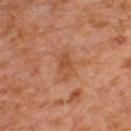Case summary:
* notes · total-body-photography surveillance lesion; no biopsy
* tile lighting · cross-polarized illumination
* lesion diameter · ≈3.5 mm
* patient · male, about 30 years old
* anatomic site · the leg
* TBP lesion metrics · a lesion color around L≈49 a*≈24 b*≈35 in CIELAB and a lesion-to-skin contrast of about 6 (normalized; higher = more distinct); border irregularity of about 2.5 on a 0–10 scale, a within-lesion color-variation index near 2.5/10, and radial color variation of about 0.5
* acquisition · 15 mm crop, total-body photography